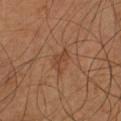Impression:
Part of a total-body skin-imaging series; this lesion was reviewed on a skin check and was not flagged for biopsy.
Image and clinical context:
About 3.5 mm across. A close-up tile cropped from a whole-body skin photograph, about 15 mm across. A male patient aged 53–57. The lesion is on the right forearm. The tile uses cross-polarized illumination.